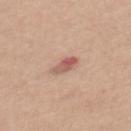biopsy_status: not biopsied; imaged during a skin examination
lighting: white-light
patient:
  sex: female
  age_approx: 35
site: back
image:
  source: total-body photography crop
  field_of_view_mm: 15
lesion_size:
  long_diameter_mm_approx: 3.0